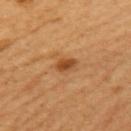This lesion was catalogued during total-body skin photography and was not selected for biopsy.
An algorithmic analysis of the crop reported a lesion area of about 3 mm², a shape eccentricity near 0.75, and two-axis asymmetry of about 0.25. And it measured an average lesion color of about L≈48 a*≈26 b*≈41 (CIELAB), roughly 12 lightness units darker than nearby skin, and a normalized lesion–skin contrast near 9. The analysis additionally found a color-variation rating of about 2.5/10 and peripheral color asymmetry of about 1. The analysis additionally found a detector confidence of about 100 out of 100 that the crop contains a lesion.
Cropped from a total-body skin-imaging series; the visible field is about 15 mm.
A female subject, roughly 55 years of age.
Captured under cross-polarized illumination.
The lesion is on the left upper arm.